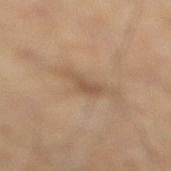Notes:
- workup — no biopsy performed (imaged during a skin exam)
- location — the leg
- patient — male, in their mid- to late 40s
- lesion size — about 3 mm
- imaging modality — 15 mm crop, total-body photography